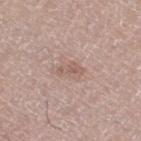This lesion was catalogued during total-body skin photography and was not selected for biopsy. A male patient, aged 63–67. About 3 mm across. This is a white-light tile. The lesion is located on the leg. A region of skin cropped from a whole-body photographic capture, roughly 15 mm wide.